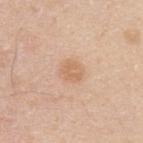  biopsy_status: not biopsied; imaged during a skin examination
  lighting: white-light
  patient:
    sex: male
    age_approx: 30
  site: upper back
  image:
    source: total-body photography crop
    field_of_view_mm: 15